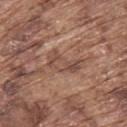Case summary:
– workup · catalogued during a skin exam; not biopsied
– site · the back
– automated lesion analysis · a shape eccentricity near 0.85; a lesion color around L≈48 a*≈19 b*≈26 in CIELAB, roughly 8 lightness units darker than nearby skin, and a normalized lesion–skin contrast near 6; a nevus-likeness score of about 0/100 and a detector confidence of about 55 out of 100 that the crop contains a lesion
– lesion size · ~4 mm (longest diameter)
– tile lighting · white-light
– image · ~15 mm crop, total-body skin-cancer survey
– patient · male, roughly 75 years of age